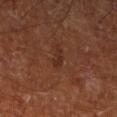Recorded during total-body skin imaging; not selected for excision or biopsy. From the left lower leg. The patient is a male in their mid-60s. Automated tile analysis of the lesion measured an average lesion color of about L≈27 a*≈21 b*≈25 (CIELAB), a lesion–skin lightness drop of about 6, and a normalized lesion–skin contrast near 6. A region of skin cropped from a whole-body photographic capture, roughly 15 mm wide. The tile uses cross-polarized illumination. Measured at roughly 2.5 mm in maximum diameter.